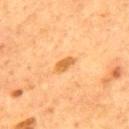Q: Was this lesion biopsied?
A: imaged on a skin check; not biopsied
Q: Lesion location?
A: the back
Q: What kind of image is this?
A: ~15 mm crop, total-body skin-cancer survey
Q: Who is the patient?
A: male, about 55 years old